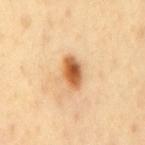Findings:
– workup — catalogued during a skin exam; not biopsied
– anatomic site — the back
– patient — male, aged 38–42
– TBP lesion metrics — border irregularity of about 2 on a 0–10 scale, a color-variation rating of about 6.5/10, and peripheral color asymmetry of about 1.5; a nevus-likeness score of about 100/100 and a detector confidence of about 100 out of 100 that the crop contains a lesion
– size — ≈3.5 mm
– image — total-body-photography crop, ~15 mm field of view